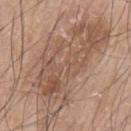Q: Is there a histopathology result?
A: imaged on a skin check; not biopsied
Q: What did automated image analysis measure?
A: an average lesion color of about L≈55 a*≈17 b*≈28 (CIELAB), roughly 9 lightness units darker than nearby skin, and a normalized lesion–skin contrast near 6; a within-lesion color-variation index near 6/10; a classifier nevus-likeness of about 5/100 and a detector confidence of about 95 out of 100 that the crop contains a lesion
Q: Lesion location?
A: the left upper arm
Q: How was this image acquired?
A: ~15 mm crop, total-body skin-cancer survey
Q: What lighting was used for the tile?
A: white-light illumination
Q: How large is the lesion?
A: ~13.5 mm (longest diameter)
Q: What are the patient's age and sex?
A: male, in their mid-70s